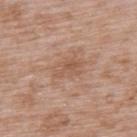| key | value |
|---|---|
| notes | no biopsy performed (imaged during a skin exam) |
| lighting | white-light illumination |
| body site | the upper back |
| lesion diameter | ≈4 mm |
| patient | male, aged 48–52 |
| imaging modality | ~15 mm tile from a whole-body skin photo |
| image-analysis metrics | a lesion area of about 7 mm², a shape eccentricity near 0.8, and a symmetry-axis asymmetry near 0.2; border irregularity of about 2.5 on a 0–10 scale, a color-variation rating of about 3.5/10, and radial color variation of about 1.5; an automated nevus-likeness rating near 0 out of 100 and lesion-presence confidence of about 100/100 |